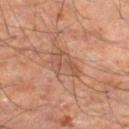workup: total-body-photography surveillance lesion; no biopsy
site: the right thigh
tile lighting: cross-polarized
image source: ~15 mm crop, total-body skin-cancer survey
TBP lesion metrics: a footprint of about 8.5 mm², an eccentricity of roughly 0.75, and two-axis asymmetry of about 0.3; lesion-presence confidence of about 100/100
size: ~4 mm (longest diameter)
subject: male, approximately 55 years of age